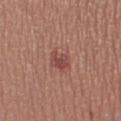Notes:
- follow-up · catalogued during a skin exam; not biopsied
- imaging modality · ~15 mm tile from a whole-body skin photo
- automated metrics · an area of roughly 5 mm², an outline eccentricity of about 0.4 (0 = round, 1 = elongated), and a symmetry-axis asymmetry near 0.3; a classifier nevus-likeness of about 85/100 and a lesion-detection confidence of about 100/100
- lesion size · ~2.5 mm (longest diameter)
- illumination · white-light
- body site · the right thigh
- subject · female, aged approximately 35